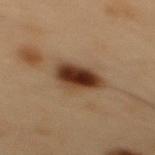Impression:
Imaged during a routine full-body skin examination; the lesion was not biopsied and no histopathology is available.
Clinical summary:
The lesion's longest dimension is about 4 mm. A 15 mm crop from a total-body photograph taken for skin-cancer surveillance. A male subject, aged 53–57. The lesion is on the back.